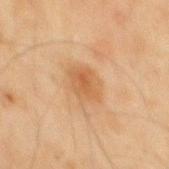This lesion was catalogued during total-body skin photography and was not selected for biopsy.
From the back.
Captured under cross-polarized illumination.
A 15 mm close-up tile from a total-body photography series done for melanoma screening.
The patient is a male approximately 45 years of age.
Measured at roughly 4 mm in maximum diameter.